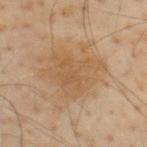site: the upper back | image: ~15 mm crop, total-body skin-cancer survey | subject: male, aged 53–57.